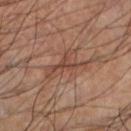The lesion was photographed on a routine skin check and not biopsied; there is no pathology result. Automated image analysis of the tile measured lesion-presence confidence of about 60/100. Located on the left lower leg. The tile uses cross-polarized illumination. A 15 mm close-up extracted from a 3D total-body photography capture. A male patient aged 58–62. About 5.5 mm across.